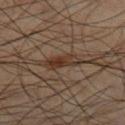Assessment: This lesion was catalogued during total-body skin photography and was not selected for biopsy. Background: The lesion is located on the leg. A male patient, approximately 60 years of age. A 15 mm close-up tile from a total-body photography series done for melanoma screening.